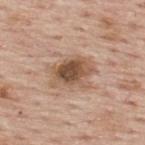Assessment: No biopsy was performed on this lesion — it was imaged during a full skin examination and was not determined to be concerning. Clinical summary: The subject is a male in their mid- to late 70s. From the upper back. This is a white-light tile. The recorded lesion diameter is about 4.5 mm. Cropped from a total-body skin-imaging series; the visible field is about 15 mm.